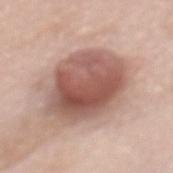Case summary:
• notes · catalogued during a skin exam; not biopsied
• location · the mid back
• lighting · white-light illumination
• lesion diameter · about 8 mm
• acquisition · 15 mm crop, total-body photography
• patient · female, aged around 60